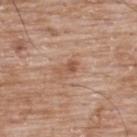Clinical impression:
Captured during whole-body skin photography for melanoma surveillance; the lesion was not biopsied.
Image and clinical context:
The lesion is located on the upper back. The total-body-photography lesion software estimated an average lesion color of about L≈54 a*≈22 b*≈31 (CIELAB), roughly 9 lightness units darker than nearby skin, and a lesion-to-skin contrast of about 6.5 (normalized; higher = more distinct). The software also gave border irregularity of about 5 on a 0–10 scale, internal color variation of about 1 on a 0–10 scale, and a peripheral color-asymmetry measure near 0.5. The software also gave an automated nevus-likeness rating near 10 out of 100. A region of skin cropped from a whole-body photographic capture, roughly 15 mm wide. Approximately 3 mm at its widest. A male subject aged around 50. Imaged with white-light lighting.